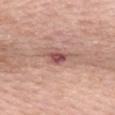biopsy status=total-body-photography surveillance lesion; no biopsy
imaging modality=~15 mm tile from a whole-body skin photo
patient=female, aged 63 to 67
image-analysis metrics=a lesion area of about 4 mm², an eccentricity of roughly 0.85, and two-axis asymmetry of about 0.25; a color-variation rating of about 4/10; a nevus-likeness score of about 55/100 and a detector confidence of about 100 out of 100 that the crop contains a lesion
anatomic site=the mid back
diameter=≈3.5 mm